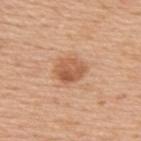On the upper back.
The recorded lesion diameter is about 3.5 mm.
This image is a 15 mm lesion crop taken from a total-body photograph.
Imaged with white-light lighting.
The patient is a female in their mid-40s.